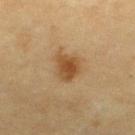acquisition=~15 mm tile from a whole-body skin photo
subject=female, aged 53–57
body site=the left lower leg